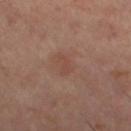Case summary:
- follow-up: catalogued during a skin exam; not biopsied
- lighting: cross-polarized
- subject: female, aged 58 to 62
- anatomic site: the left lower leg
- image source: ~15 mm tile from a whole-body skin photo
- diameter: ≈2.5 mm